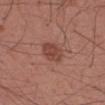notes: catalogued during a skin exam; not biopsied.